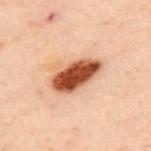Clinical impression: Captured during whole-body skin photography for melanoma surveillance; the lesion was not biopsied. Context: The lesion-visualizer software estimated an eccentricity of roughly 0.85 and two-axis asymmetry of about 0.2. Located on the upper back. Imaged with cross-polarized lighting. A male patient aged approximately 50. This image is a 15 mm lesion crop taken from a total-body photograph.